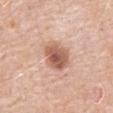Notes:
- biopsy status — total-body-photography surveillance lesion; no biopsy
- subject — male, aged around 80
- diameter — about 4 mm
- image source — ~15 mm tile from a whole-body skin photo
- illumination — white-light illumination
- anatomic site — the abdomen